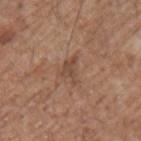The lesion was photographed on a routine skin check and not biopsied; there is no pathology result. The lesion is located on the left upper arm. A lesion tile, about 15 mm wide, cut from a 3D total-body photograph. The subject is a male approximately 70 years of age. This is a white-light tile. The lesion's longest dimension is about 3 mm.